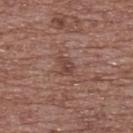  biopsy_status: not biopsied; imaged during a skin examination
  patient:
    sex: female
    age_approx: 75
  lighting: white-light
  lesion_size:
    long_diameter_mm_approx: 2.5
  site: upper back
  image:
    source: total-body photography crop
    field_of_view_mm: 15